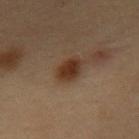<tbp_lesion>
  <biopsy_status>not biopsied; imaged during a skin examination</biopsy_status>
  <patient>
    <sex>male</sex>
    <age_approx>55</age_approx>
  </patient>
  <site>front of the torso</site>
  <lighting>cross-polarized</lighting>
  <image>
    <source>total-body photography crop</source>
    <field_of_view_mm>15</field_of_view_mm>
  </image>
</tbp_lesion>